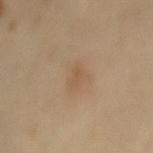The lesion was photographed on a routine skin check and not biopsied; there is no pathology result. An algorithmic analysis of the crop reported internal color variation of about 0 on a 0–10 scale and a peripheral color-asymmetry measure near 0. A female subject in their mid- to late 50s. Approximately 2 mm at its widest. This image is a 15 mm lesion crop taken from a total-body photograph. The lesion is located on the mid back. This is a cross-polarized tile.